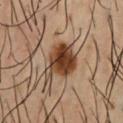Case summary:
– workup: total-body-photography surveillance lesion; no biopsy
– site: the chest
– image-analysis metrics: a mean CIELAB color near L≈40 a*≈19 b*≈30, a lesion–skin lightness drop of about 16, and a normalized border contrast of about 12.5; a border-irregularity index near 3.5/10 and internal color variation of about 6.5 on a 0–10 scale; a nevus-likeness score of about 100/100 and lesion-presence confidence of about 100/100
– patient: male, about 55 years old
– image source: ~15 mm crop, total-body skin-cancer survey